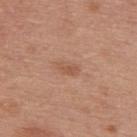Notes:
* notes — no biopsy performed (imaged during a skin exam)
* subject — male, about 30 years old
* diameter — about 2.5 mm
* body site — the upper back
* acquisition — 15 mm crop, total-body photography
* illumination — white-light